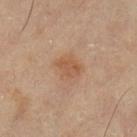Q: Was a biopsy performed?
A: total-body-photography surveillance lesion; no biopsy
Q: How large is the lesion?
A: ~3 mm (longest diameter)
Q: What is the imaging modality?
A: total-body-photography crop, ~15 mm field of view
Q: How was the tile lit?
A: cross-polarized
Q: Who is the patient?
A: female, approximately 70 years of age
Q: Lesion location?
A: the left lower leg